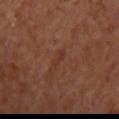Assessment:
Recorded during total-body skin imaging; not selected for excision or biopsy.
Clinical summary:
Imaged with cross-polarized lighting. The lesion is located on the chest. About 2.5 mm across. The patient is a male about 70 years old. The lesion-visualizer software estimated a border-irregularity rating of about 3.5/10, a color-variation rating of about 0/10, and peripheral color asymmetry of about 0. A lesion tile, about 15 mm wide, cut from a 3D total-body photograph.